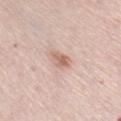Impression:
No biopsy was performed on this lesion — it was imaged during a full skin examination and was not determined to be concerning.
Background:
The lesion is located on the front of the torso. An algorithmic analysis of the crop reported a lesion area of about 4 mm² and a shape eccentricity near 0.75. It also reported an automated nevus-likeness rating near 55 out of 100 and a lesion-detection confidence of about 100/100. The tile uses white-light illumination. Cropped from a total-body skin-imaging series; the visible field is about 15 mm. The subject is a female about 70 years old. The recorded lesion diameter is about 3 mm.